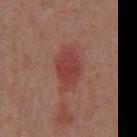Impression: The lesion was photographed on a routine skin check and not biopsied; there is no pathology result. Acquisition and patient details: A male patient aged 53–57. This is a white-light tile. A close-up tile cropped from a whole-body skin photograph, about 15 mm across. The lesion is located on the chest. About 5 mm across.